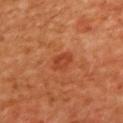<tbp_lesion>
  <biopsy_status>not biopsied; imaged during a skin examination</biopsy_status>
  <image>
    <source>total-body photography crop</source>
    <field_of_view_mm>15</field_of_view_mm>
  </image>
  <site>upper back</site>
  <lesion_size>
    <long_diameter_mm_approx>2.5</long_diameter_mm_approx>
  </lesion_size>
  <lighting>cross-polarized</lighting>
  <patient>
    <sex>female</sex>
    <age_approx>40</age_approx>
  </patient>
</tbp_lesion>